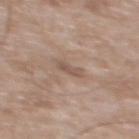biopsy_status: not biopsied; imaged during a skin examination
patient:
  sex: male
  age_approx: 50
lesion_size:
  long_diameter_mm_approx: 2.5
site: back
automated_metrics:
  area_mm2_approx: 2.5
  eccentricity: 0.9
  shape_asymmetry: 0.3
  cielab_L: 53
  cielab_a: 16
  cielab_b: 25
  vs_skin_darker_L: 8.0
  vs_skin_contrast_norm: 6.0
  color_variation_0_10: 0.0
  peripheral_color_asymmetry: 0.0
image:
  source: total-body photography crop
  field_of_view_mm: 15